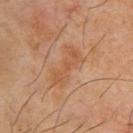Recorded during total-body skin imaging; not selected for excision or biopsy. The subject is a male in their mid-60s. Located on the back. A 15 mm close-up extracted from a 3D total-body photography capture.